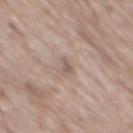Captured during whole-body skin photography for melanoma surveillance; the lesion was not biopsied.
The lesion's longest dimension is about 2.5 mm.
A 15 mm close-up tile from a total-body photography series done for melanoma screening.
The tile uses white-light illumination.
The lesion is on the mid back.
The patient is a male aged around 70.